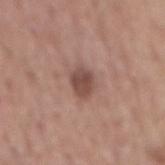Clinical impression:
The lesion was tiled from a total-body skin photograph and was not biopsied.
Acquisition and patient details:
From the back. Measured at roughly 3.5 mm in maximum diameter. A 15 mm close-up tile from a total-body photography series done for melanoma screening. A male patient, aged 53 to 57.